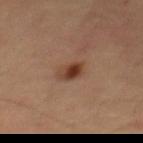biopsy status=imaged on a skin check; not biopsied
image=15 mm crop, total-body photography
lighting=cross-polarized illumination
subject=male, aged 63 to 67
anatomic site=the mid back